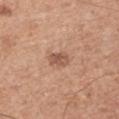{"biopsy_status": "not biopsied; imaged during a skin examination", "patient": {"sex": "male", "age_approx": 55}, "image": {"source": "total-body photography crop", "field_of_view_mm": 15}, "automated_metrics": {"area_mm2_approx": 4.5, "eccentricity": 0.75, "shape_asymmetry": 0.25, "vs_skin_darker_L": 10.0, "border_irregularity_0_10": 2.0, "color_variation_0_10": 3.0, "peripheral_color_asymmetry": 1.0}, "lighting": "white-light", "site": "right upper arm", "lesion_size": {"long_diameter_mm_approx": 2.5}}